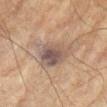Part of a total-body skin-imaging series; this lesion was reviewed on a skin check and was not flagged for biopsy.
Longest diameter approximately 4.5 mm.
The tile uses cross-polarized illumination.
Located on the left upper arm.
A 15 mm crop from a total-body photograph taken for skin-cancer surveillance.
The subject is a male aged approximately 75.